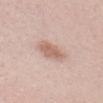biopsy status: catalogued during a skin exam; not biopsied
image-analysis metrics: a lesion area of about 8 mm², an eccentricity of roughly 0.85, and a shape-asymmetry score of about 0.2 (0 = symmetric); border irregularity of about 2.5 on a 0–10 scale and a peripheral color-asymmetry measure near 1
lesion diameter: ≈4.5 mm
location: the head or neck
image source: ~15 mm crop, total-body skin-cancer survey
tile lighting: white-light illumination
patient: female, aged around 20